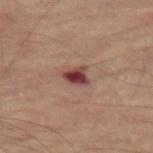Clinical impression:
No biopsy was performed on this lesion — it was imaged during a full skin examination and was not determined to be concerning.
Context:
This is a cross-polarized tile. A male subject approximately 65 years of age. The lesion is located on the left thigh. This image is a 15 mm lesion crop taken from a total-body photograph. The lesion's longest dimension is about 2.5 mm.